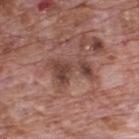notes — no biopsy performed (imaged during a skin exam) | site — the upper back | patient — male, aged 68 to 72 | imaging modality — ~15 mm crop, total-body skin-cancer survey.